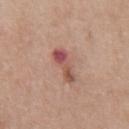No biopsy was performed on this lesion — it was imaged during a full skin examination and was not determined to be concerning. Cropped from a total-body skin-imaging series; the visible field is about 15 mm. On the abdomen. Imaged with white-light lighting. Measured at roughly 4.5 mm in maximum diameter. A male patient, in their 50s. An algorithmic analysis of the crop reported an area of roughly 7 mm² and a shape eccentricity near 0.95. And it measured an average lesion color of about L≈53 a*≈25 b*≈25 (CIELAB), roughly 11 lightness units darker than nearby skin, and a normalized border contrast of about 7.5. And it measured a border-irregularity index near 3/10, a color-variation rating of about 10/10, and a peripheral color-asymmetry measure near 3.5. It also reported a classifier nevus-likeness of about 0/100 and a lesion-detection confidence of about 100/100.